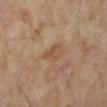| feature | finding |
|---|---|
| notes | no biopsy performed (imaged during a skin exam) |
| acquisition | total-body-photography crop, ~15 mm field of view |
| size | ≈3 mm |
| illumination | cross-polarized illumination |
| subject | female, roughly 75 years of age |
| location | the right lower leg |